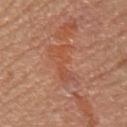Notes:
– notes: no biopsy performed (imaged during a skin exam)
– diameter: ~5 mm (longest diameter)
– patient: female, aged approximately 60
– body site: the right forearm
– imaging modality: ~15 mm crop, total-body skin-cancer survey
– TBP lesion metrics: lesion-presence confidence of about 100/100
– tile lighting: white-light